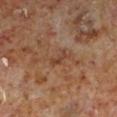Recorded during total-body skin imaging; not selected for excision or biopsy. The tile uses cross-polarized illumination. A male patient aged 58–62. A 15 mm close-up extracted from a 3D total-body photography capture. From the left lower leg. About 3 mm across. The lesion-visualizer software estimated a footprint of about 2.5 mm² and an eccentricity of roughly 0.9. It also reported an automated nevus-likeness rating near 0 out of 100 and a detector confidence of about 100 out of 100 that the crop contains a lesion.